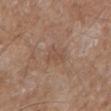follow-up=total-body-photography surveillance lesion; no biopsy | image=~15 mm tile from a whole-body skin photo | tile lighting=white-light illumination | body site=the arm | patient=male, approximately 60 years of age | lesion size=≈2.5 mm.